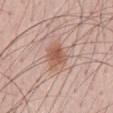The lesion was photographed on a routine skin check and not biopsied; there is no pathology result. Automated tile analysis of the lesion measured a border-irregularity index near 2/10, internal color variation of about 3.5 on a 0–10 scale, and peripheral color asymmetry of about 1. And it measured an automated nevus-likeness rating near 90 out of 100 and a detector confidence of about 100 out of 100 that the crop contains a lesion. A male subject, aged 53–57. A region of skin cropped from a whole-body photographic capture, roughly 15 mm wide. The tile uses white-light illumination. On the abdomen.